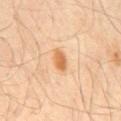biopsy status: catalogued during a skin exam; not biopsied | automated metrics: a lesion area of about 4 mm², an eccentricity of roughly 0.75, and a shape-asymmetry score of about 0.25 (0 = symmetric); an automated nevus-likeness rating near 100 out of 100 and a detector confidence of about 100 out of 100 that the crop contains a lesion | tile lighting: cross-polarized | patient: male, about 65 years old | site: the mid back | lesion size: about 3 mm | acquisition: total-body-photography crop, ~15 mm field of view.